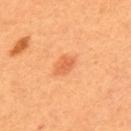This lesion was catalogued during total-body skin photography and was not selected for biopsy. The tile uses cross-polarized illumination. Cropped from a total-body skin-imaging series; the visible field is about 15 mm. Approximately 3 mm at its widest. The subject is a female aged approximately 50.